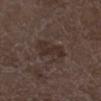Recorded during total-body skin imaging; not selected for excision or biopsy.
A male patient roughly 75 years of age.
The total-body-photography lesion software estimated a shape eccentricity near 0.8 and two-axis asymmetry of about 0.4.
From the right lower leg.
A region of skin cropped from a whole-body photographic capture, roughly 15 mm wide.
Imaged with white-light lighting.
About 4 mm across.